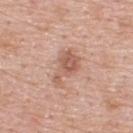Q: Was this lesion biopsied?
A: total-body-photography surveillance lesion; no biopsy
Q: How was the tile lit?
A: white-light illumination
Q: Patient demographics?
A: male, in their mid-50s
Q: What is the imaging modality?
A: total-body-photography crop, ~15 mm field of view
Q: What is the lesion's diameter?
A: ~4 mm (longest diameter)
Q: Where on the body is the lesion?
A: the back